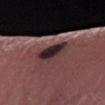Notes:
- notes: no biopsy performed (imaged during a skin exam)
- subject: female, aged 38 to 42
- body site: the right forearm
- image: ~15 mm tile from a whole-body skin photo
- size: ~3.5 mm (longest diameter)
- automated lesion analysis: a mean CIELAB color near L≈27 a*≈18 b*≈12